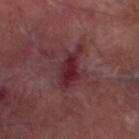Recorded during total-body skin imaging; not selected for excision or biopsy. An algorithmic analysis of the crop reported an average lesion color of about L≈27 a*≈26 b*≈16 (CIELAB), a lesion–skin lightness drop of about 10, and a normalized border contrast of about 9.5. It also reported a nevus-likeness score of about 0/100. The subject is a male roughly 70 years of age. A lesion tile, about 15 mm wide, cut from a 3D total-body photograph. Located on the left lower leg.